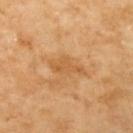Assessment:
No biopsy was performed on this lesion — it was imaged during a full skin examination and was not determined to be concerning.
Clinical summary:
From the left upper arm. A female patient aged approximately 70. The recorded lesion diameter is about 4 mm. This is a cross-polarized tile. The total-body-photography lesion software estimated an average lesion color of about L≈60 a*≈23 b*≈44 (CIELAB) and roughly 8 lightness units darker than nearby skin. A region of skin cropped from a whole-body photographic capture, roughly 15 mm wide.